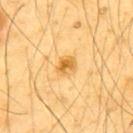| key | value |
|---|---|
| notes | catalogued during a skin exam; not biopsied |
| lighting | cross-polarized |
| subject | male, in their mid- to late 60s |
| anatomic site | the upper back |
| image source | ~15 mm tile from a whole-body skin photo |
| lesion diameter | ≈2.5 mm |